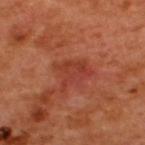Case summary:
• size — ≈4 mm
• anatomic site — the upper back
• image-analysis metrics — a lesion–skin lightness drop of about 7 and a lesion-to-skin contrast of about 5.5 (normalized; higher = more distinct); a nevus-likeness score of about 0/100 and a lesion-detection confidence of about 100/100
• subject — male, about 50 years old
• imaging modality — ~15 mm tile from a whole-body skin photo
• lighting — cross-polarized illumination